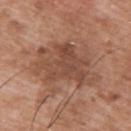image = total-body-photography crop, ~15 mm field of view | anatomic site = the upper back | lesion size = about 7.5 mm | patient = male, roughly 50 years of age | lighting = white-light illumination.